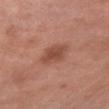| key | value |
|---|---|
| biopsy status | imaged on a skin check; not biopsied |
| subject | male, aged 53 to 57 |
| lesion diameter | about 3.5 mm |
| lighting | white-light |
| location | the left upper arm |
| acquisition | ~15 mm tile from a whole-body skin photo |